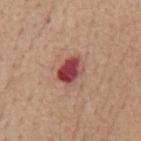biopsy status: imaged on a skin check; not biopsied | subject: male, in their mid- to late 60s | acquisition: 15 mm crop, total-body photography | site: the back | illumination: white-light illumination | diameter: ≈3.5 mm.